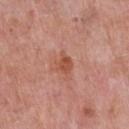Q: Was this lesion biopsied?
A: no biopsy performed (imaged during a skin exam)
Q: What did automated image analysis measure?
A: a lesion area of about 4 mm² and an eccentricity of roughly 0.65; a lesion-to-skin contrast of about 7.5 (normalized; higher = more distinct)
Q: What is the imaging modality?
A: ~15 mm tile from a whole-body skin photo
Q: Illumination type?
A: white-light
Q: Lesion size?
A: about 2.5 mm
Q: Lesion location?
A: the chest
Q: Patient demographics?
A: male, aged around 50